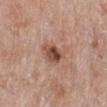Q: Is there a histopathology result?
A: catalogued during a skin exam; not biopsied
Q: Automated lesion metrics?
A: an average lesion color of about L≈49 a*≈21 b*≈27 (CIELAB), a lesion–skin lightness drop of about 13, and a normalized border contrast of about 9
Q: How was the tile lit?
A: white-light
Q: How large is the lesion?
A: about 3.5 mm
Q: How was this image acquired?
A: ~15 mm tile from a whole-body skin photo
Q: What are the patient's age and sex?
A: female, about 55 years old
Q: What is the anatomic site?
A: the leg